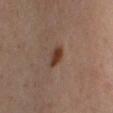Imaged during a routine full-body skin examination; the lesion was not biopsied and no histopathology is available. Approximately 3 mm at its widest. A lesion tile, about 15 mm wide, cut from a 3D total-body photograph. A female subject, aged 48 to 52. The lesion is on the right thigh.